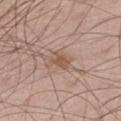| feature | finding |
|---|---|
| notes | total-body-photography surveillance lesion; no biopsy |
| location | the right thigh |
| lighting | white-light illumination |
| image | 15 mm crop, total-body photography |
| subject | male, about 65 years old |
| image-analysis metrics | an area of roughly 4.5 mm² and an outline eccentricity of about 0.65 (0 = round, 1 = elongated); a lesion color around L≈54 a*≈18 b*≈28 in CIELAB and a normalized border contrast of about 6.5; an automated nevus-likeness rating near 25 out of 100 and a detector confidence of about 100 out of 100 that the crop contains a lesion |